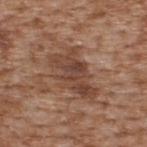– notes — no biopsy performed (imaged during a skin exam)
– acquisition — ~15 mm tile from a whole-body skin photo
– anatomic site — the upper back
– patient — male, aged 63–67